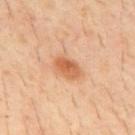The lesion-visualizer software estimated a border-irregularity index near 2/10.
The lesion is on the mid back.
A 15 mm close-up tile from a total-body photography series done for melanoma screening.
The subject is a male roughly 30 years of age.
Captured under cross-polarized illumination.